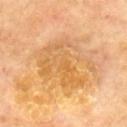Clinical impression: The lesion was tiled from a total-body skin photograph and was not biopsied. Acquisition and patient details: Cropped from a whole-body photographic skin survey; the tile spans about 15 mm. On the upper back. Measured at roughly 8 mm in maximum diameter. This is a cross-polarized tile. The subject is a male aged 68–72. The lesion-visualizer software estimated a lesion area of about 28 mm², a shape eccentricity near 0.65, and two-axis asymmetry of about 0.35. The analysis additionally found a mean CIELAB color near L≈64 a*≈21 b*≈45, about 8 CIELAB-L* units darker than the surrounding skin, and a lesion-to-skin contrast of about 5.5 (normalized; higher = more distinct). And it measured a classifier nevus-likeness of about 0/100 and a lesion-detection confidence of about 100/100.